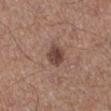The patient is a male in their mid- to late 60s.
This is a white-light tile.
About 2.5 mm across.
Located on the leg.
A 15 mm close-up extracted from a 3D total-body photography capture.